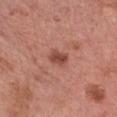follow-up = catalogued during a skin exam; not biopsied
tile lighting = white-light illumination
site = the chest
acquisition = ~15 mm crop, total-body skin-cancer survey
subject = female, aged 58–62
lesion size = ~2.5 mm (longest diameter)